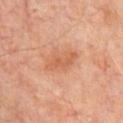Imaged during a routine full-body skin examination; the lesion was not biopsied and no histopathology is available. The tile uses cross-polarized illumination. The subject is a male about 60 years old. Located on the chest. Automated image analysis of the tile measured a footprint of about 6.5 mm², a shape eccentricity near 0.85, and a symmetry-axis asymmetry near 0.35. It also reported a nevus-likeness score of about 5/100 and lesion-presence confidence of about 100/100. A 15 mm close-up extracted from a 3D total-body photography capture. Longest diameter approximately 4 mm.